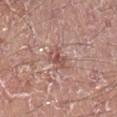Q: Was this lesion biopsied?
A: no biopsy performed (imaged during a skin exam)
Q: Illumination type?
A: white-light illumination
Q: Lesion location?
A: the right lower leg
Q: Lesion size?
A: ~3.5 mm (longest diameter)
Q: What are the patient's age and sex?
A: male, aged 18 to 22
Q: Automated lesion metrics?
A: a border-irregularity index near 4/10, internal color variation of about 4 on a 0–10 scale, and radial color variation of about 1
Q: How was this image acquired?
A: ~15 mm tile from a whole-body skin photo